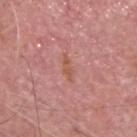  biopsy_status: not biopsied; imaged during a skin examination
  lighting: white-light
  patient:
    sex: male
    age_approx: 75
  site: head or neck
  automated_metrics:
    border_irregularity_0_10: 4.0
    color_variation_0_10: 0.0
    peripheral_color_asymmetry: 0.0
    lesion_detection_confidence_0_100: 95
  image:
    source: total-body photography crop
    field_of_view_mm: 15
  lesion_size:
    long_diameter_mm_approx: 3.0